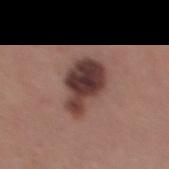The lesion was photographed on a routine skin check and not biopsied; there is no pathology result. Cropped from a total-body skin-imaging series; the visible field is about 15 mm. Automated image analysis of the tile measured a footprint of about 16 mm², an eccentricity of roughly 0.8, and two-axis asymmetry of about 0.3. It also reported a mean CIELAB color near L≈38 a*≈20 b*≈20, a lesion–skin lightness drop of about 16, and a normalized border contrast of about 12.5. The software also gave a border-irregularity rating of about 3/10 and internal color variation of about 6.5 on a 0–10 scale. And it measured a nevus-likeness score of about 25/100. The subject is a male roughly 40 years of age. Located on the back. The recorded lesion diameter is about 5.5 mm.